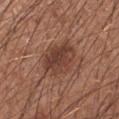Impression:
Part of a total-body skin-imaging series; this lesion was reviewed on a skin check and was not flagged for biopsy.
Background:
Measured at roughly 4.5 mm in maximum diameter. From the left forearm. A lesion tile, about 15 mm wide, cut from a 3D total-body photograph. Captured under white-light illumination. A male patient, roughly 30 years of age. The lesion-visualizer software estimated a lesion area of about 12 mm², an eccentricity of roughly 0.6, and a symmetry-axis asymmetry near 0.2. And it measured a lesion color around L≈40 a*≈21 b*≈26 in CIELAB and a lesion–skin lightness drop of about 10. The software also gave border irregularity of about 2 on a 0–10 scale and internal color variation of about 5 on a 0–10 scale.